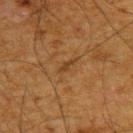Impression: Imaged during a routine full-body skin examination; the lesion was not biopsied and no histopathology is available. Acquisition and patient details: The recorded lesion diameter is about 2.5 mm. A male subject roughly 65 years of age. The lesion is located on the upper back. Imaged with cross-polarized lighting. A 15 mm close-up extracted from a 3D total-body photography capture.